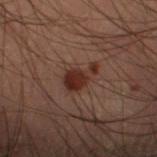The lesion was photographed on a routine skin check and not biopsied; there is no pathology result. Approximately 4 mm at its widest. This is a cross-polarized tile. A male subject aged approximately 55. The lesion is on the left thigh. This image is a 15 mm lesion crop taken from a total-body photograph.